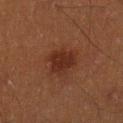Impression: Captured during whole-body skin photography for melanoma surveillance; the lesion was not biopsied. Context: Cropped from a whole-body photographic skin survey; the tile spans about 15 mm. Measured at roughly 4 mm in maximum diameter. Located on the right lower leg. The tile uses cross-polarized illumination. An algorithmic analysis of the crop reported a lesion area of about 8.5 mm² and a symmetry-axis asymmetry near 0.25. The analysis additionally found a border-irregularity index near 2.5/10 and a peripheral color-asymmetry measure near 0.5. And it measured a nevus-likeness score of about 50/100 and a lesion-detection confidence of about 100/100. A male subject, aged 38–42.